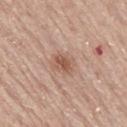The lesion was photographed on a routine skin check and not biopsied; there is no pathology result. The lesion-visualizer software estimated a lesion area of about 6.5 mm² and an eccentricity of roughly 0.65. The software also gave a lesion color around L≈56 a*≈20 b*≈29 in CIELAB, roughly 9 lightness units darker than nearby skin, and a lesion-to-skin contrast of about 6.5 (normalized; higher = more distinct). Longest diameter approximately 3.5 mm. Cropped from a whole-body photographic skin survey; the tile spans about 15 mm. The patient is a male aged around 80. The lesion is located on the mid back.